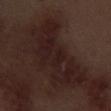biopsy status — no biopsy performed (imaged during a skin exam); patient — male, in their 70s; diameter — about 9.5 mm; imaging modality — 15 mm crop, total-body photography; tile lighting — white-light illumination; anatomic site — the leg.